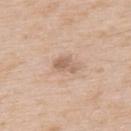This image is a 15 mm lesion crop taken from a total-body photograph.
A male subject, aged 63 to 67.
Located on the back.
Measured at roughly 2.5 mm in maximum diameter.
The tile uses white-light illumination.